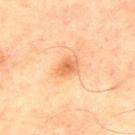Recorded during total-body skin imaging; not selected for excision or biopsy.
A 15 mm close-up extracted from a 3D total-body photography capture.
A male subject, in their mid-60s.
The lesion-visualizer software estimated a footprint of about 4.5 mm², an eccentricity of roughly 0.65, and two-axis asymmetry of about 0.15. And it measured about 9 CIELAB-L* units darker than the surrounding skin and a normalized border contrast of about 7. The analysis additionally found a classifier nevus-likeness of about 70/100.
Approximately 3 mm at its widest.
Located on the chest.
The tile uses cross-polarized illumination.